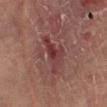Clinical impression:
The lesion was photographed on a routine skin check and not biopsied; there is no pathology result.
Context:
A male subject approximately 55 years of age. An algorithmic analysis of the crop reported an area of roughly 8 mm², an outline eccentricity of about 0.9 (0 = round, 1 = elongated), and a shape-asymmetry score of about 0.6 (0 = symmetric). The analysis additionally found an average lesion color of about L≈32 a*≈23 b*≈18 (CIELAB), about 8 CIELAB-L* units darker than the surrounding skin, and a normalized lesion–skin contrast near 7.5. And it measured border irregularity of about 7.5 on a 0–10 scale, a within-lesion color-variation index near 5/10, and peripheral color asymmetry of about 1. From the left lower leg. This image is a 15 mm lesion crop taken from a total-body photograph. Captured under cross-polarized illumination.